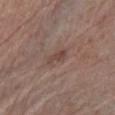follow-up = catalogued during a skin exam; not biopsied | imaging modality = 15 mm crop, total-body photography | patient = male, approximately 70 years of age | lesion size = about 2.5 mm | anatomic site = the leg.